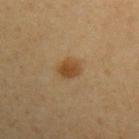Assessment: The lesion was photographed on a routine skin check and not biopsied; there is no pathology result. Context: The subject is a male aged around 55. The lesion's longest dimension is about 2.5 mm. This image is a 15 mm lesion crop taken from a total-body photograph. Located on the left upper arm.